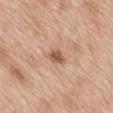notes — imaged on a skin check; not biopsied.